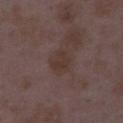biopsy status — total-body-photography surveillance lesion; no biopsy | lesion diameter — about 2.5 mm | image — ~15 mm tile from a whole-body skin photo | subject — female, about 35 years old | body site — the right thigh | illumination — white-light illumination.